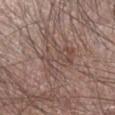Image and clinical context:
Captured under white-light illumination. The lesion's longest dimension is about 4.5 mm. Cropped from a whole-body photographic skin survey; the tile spans about 15 mm. A male patient aged approximately 60. Automated image analysis of the tile measured a shape eccentricity near 0.5 and a shape-asymmetry score of about 0.35 (0 = symmetric). It also reported a mean CIELAB color near L≈48 a*≈16 b*≈22, about 6 CIELAB-L* units darker than the surrounding skin, and a lesion-to-skin contrast of about 4.5 (normalized; higher = more distinct). It also reported a nevus-likeness score of about 0/100. From the arm.